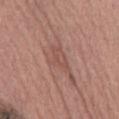| field | value |
|---|---|
| follow-up | imaged on a skin check; not biopsied |
| site | the abdomen |
| image source | total-body-photography crop, ~15 mm field of view |
| automated metrics | a lesion area of about 6 mm², an eccentricity of roughly 0.85, and a symmetry-axis asymmetry near 0.4; a lesion color around L≈51 a*≈22 b*≈25 in CIELAB, roughly 7 lightness units darker than nearby skin, and a lesion-to-skin contrast of about 5 (normalized; higher = more distinct); a classifier nevus-likeness of about 0/100 and a lesion-detection confidence of about 65/100 |
| lesion size | ≈4 mm |
| patient | male, in their mid-70s |
| tile lighting | white-light illumination |